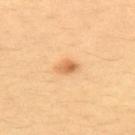- tile lighting — cross-polarized
- subject — female, aged approximately 35
- automated lesion analysis — a lesion area of about 3.5 mm² and a shape eccentricity near 0.75; a mean CIELAB color near L≈67 a*≈23 b*≈43 and about 12 CIELAB-L* units darker than the surrounding skin
- image — 15 mm crop, total-body photography
- location — the upper back
- size — about 2.5 mm